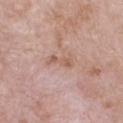The lesion was tiled from a total-body skin photograph and was not biopsied.
Approximately 3.5 mm at its widest.
Automated tile analysis of the lesion measured an area of roughly 3 mm² and a shape-asymmetry score of about 0.4 (0 = symmetric). The software also gave a lesion–skin lightness drop of about 8 and a normalized lesion–skin contrast near 6. The software also gave a classifier nevus-likeness of about 0/100 and lesion-presence confidence of about 100/100.
A male subject roughly 50 years of age.
The lesion is on the chest.
A 15 mm close-up tile from a total-body photography series done for melanoma screening.
Imaged with white-light lighting.